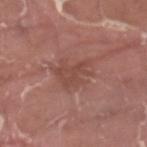biopsy status: imaged on a skin check; not biopsied
anatomic site: the left thigh
lesion diameter: about 5 mm
patient: male, roughly 40 years of age
illumination: white-light
image-analysis metrics: a mean CIELAB color near L≈47 a*≈23 b*≈25 and about 7 CIELAB-L* units darker than the surrounding skin; an automated nevus-likeness rating near 0 out of 100
image source: ~15 mm tile from a whole-body skin photo